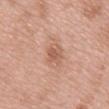follow-up = no biopsy performed (imaged during a skin exam)
lighting = white-light illumination
lesion size = ~3.5 mm (longest diameter)
automated metrics = an eccentricity of roughly 0.85 and two-axis asymmetry of about 0.25; a border-irregularity rating of about 3/10, a color-variation rating of about 2.5/10, and a peripheral color-asymmetry measure near 0.5; an automated nevus-likeness rating near 5 out of 100
location = the mid back
patient = female, aged 68 to 72
image source = total-body-photography crop, ~15 mm field of view